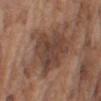No biopsy was performed on this lesion — it was imaged during a full skin examination and was not determined to be concerning. The lesion is located on the right upper arm. Automated image analysis of the tile measured a lesion color around L≈43 a*≈18 b*≈26 in CIELAB and a lesion–skin lightness drop of about 9. Longest diameter approximately 8.5 mm. This image is a 15 mm lesion crop taken from a total-body photograph. A male subject about 75 years old. Imaged with white-light lighting.